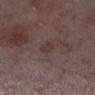{
  "biopsy_status": "not biopsied; imaged during a skin examination",
  "automated_metrics": {
    "area_mm2_approx": 2.0,
    "eccentricity": 0.8,
    "shape_asymmetry": 0.45,
    "border_irregularity_0_10": 4.0,
    "color_variation_0_10": 0.0,
    "nevus_likeness_0_100": 0,
    "lesion_detection_confidence_0_100": 100
  },
  "lesion_size": {
    "long_diameter_mm_approx": 2.0
  },
  "patient": {
    "sex": "female",
    "age_approx": 35
  },
  "lighting": "white-light",
  "image": {
    "source": "total-body photography crop",
    "field_of_view_mm": 15
  },
  "site": "right lower leg"
}